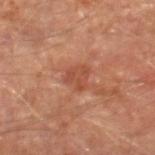Q: Was a biopsy performed?
A: imaged on a skin check; not biopsied
Q: What did automated image analysis measure?
A: border irregularity of about 3.5 on a 0–10 scale, a color-variation rating of about 2/10, and radial color variation of about 0.5
Q: How was the tile lit?
A: cross-polarized illumination
Q: Who is the patient?
A: male, roughly 65 years of age
Q: What kind of image is this?
A: 15 mm crop, total-body photography
Q: Where on the body is the lesion?
A: the leg
Q: How large is the lesion?
A: ~2.5 mm (longest diameter)